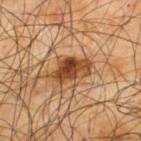Imaged during a routine full-body skin examination; the lesion was not biopsied and no histopathology is available. Automated tile analysis of the lesion measured a footprint of about 10 mm², a shape eccentricity near 0.85, and a symmetry-axis asymmetry near 0.25. The analysis additionally found a mean CIELAB color near L≈44 a*≈22 b*≈35, about 15 CIELAB-L* units darker than the surrounding skin, and a lesion-to-skin contrast of about 11 (normalized; higher = more distinct). It also reported a nevus-likeness score of about 90/100 and lesion-presence confidence of about 100/100. A male patient aged around 65. The lesion's longest dimension is about 5 mm. Cropped from a whole-body photographic skin survey; the tile spans about 15 mm. The lesion is located on the upper back.